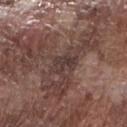The subject is a male aged 73–77. The total-body-photography lesion software estimated a lesion area of about 3.5 mm² and two-axis asymmetry of about 0.4. The software also gave a border-irregularity rating of about 4.5/10, a color-variation rating of about 1/10, and peripheral color asymmetry of about 0.5. From the right forearm. Cropped from a total-body skin-imaging series; the visible field is about 15 mm.